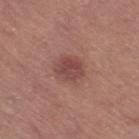<lesion>
<biopsy_status>not biopsied; imaged during a skin examination</biopsy_status>
<automated_metrics>
  <area_mm2_approx>6.5</area_mm2_approx>
  <eccentricity>0.55</eccentricity>
  <cielab_L>45</cielab_L>
  <cielab_a>24</cielab_a>
  <cielab_b>22</cielab_b>
  <vs_skin_darker_L>9.0</vs_skin_darker_L>
  <vs_skin_contrast_norm>7.5</vs_skin_contrast_norm>
</automated_metrics>
<image>
  <source>total-body photography crop</source>
  <field_of_view_mm>15</field_of_view_mm>
</image>
<site>right thigh</site>
<lighting>white-light</lighting>
<patient>
  <sex>female</sex>
  <age_approx>60</age_approx>
</patient>
<lesion_size>
  <long_diameter_mm_approx>3.0</long_diameter_mm_approx>
</lesion_size>
</lesion>